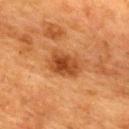This lesion was catalogued during total-body skin photography and was not selected for biopsy.
A female subject, aged around 55.
The lesion-visualizer software estimated a within-lesion color-variation index near 4/10 and radial color variation of about 1. The analysis additionally found a lesion-detection confidence of about 100/100.
On the back.
The tile uses cross-polarized illumination.
Cropped from a whole-body photographic skin survey; the tile spans about 15 mm.
Longest diameter approximately 4 mm.